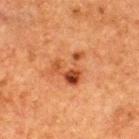Captured during whole-body skin photography for melanoma surveillance; the lesion was not biopsied.